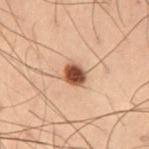notes = imaged on a skin check; not biopsied
image = total-body-photography crop, ~15 mm field of view
subject = male, approximately 55 years of age
lesion diameter = ≈2.5 mm
tile lighting = cross-polarized illumination
anatomic site = the left thigh